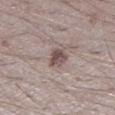Captured during whole-body skin photography for melanoma surveillance; the lesion was not biopsied. Cropped from a total-body skin-imaging series; the visible field is about 15 mm. The patient is a male aged approximately 25. The total-body-photography lesion software estimated an outline eccentricity of about 0.65 (0 = round, 1 = elongated). The analysis additionally found about 12 CIELAB-L* units darker than the surrounding skin and a normalized lesion–skin contrast near 8.5. The analysis additionally found a border-irregularity rating of about 3/10, internal color variation of about 4 on a 0–10 scale, and a peripheral color-asymmetry measure near 1.5. The recorded lesion diameter is about 3 mm. Imaged with white-light lighting. The lesion is located on the leg.